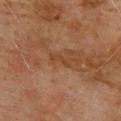follow-up = no biopsy performed (imaged during a skin exam)
patient = male, aged approximately 75
anatomic site = the upper back
image = total-body-photography crop, ~15 mm field of view